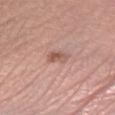This lesion was catalogued during total-body skin photography and was not selected for biopsy.
Longest diameter approximately 2.5 mm.
On the right lower leg.
Cropped from a whole-body photographic skin survey; the tile spans about 15 mm.
Imaged with white-light lighting.
A male subject aged 68 to 72.